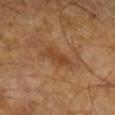Assessment: No biopsy was performed on this lesion — it was imaged during a full skin examination and was not determined to be concerning. Background: Automated image analysis of the tile measured about 7 CIELAB-L* units darker than the surrounding skin and a normalized lesion–skin contrast near 6. It also reported a border-irregularity rating of about 4/10, a within-lesion color-variation index near 2.5/10, and radial color variation of about 1. A male patient, about 65 years old. A region of skin cropped from a whole-body photographic capture, roughly 15 mm wide. This is a cross-polarized tile. The lesion's longest dimension is about 4.5 mm.